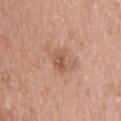biopsy status = total-body-photography surveillance lesion; no biopsy | imaging modality = 15 mm crop, total-body photography | automated lesion analysis = a nevus-likeness score of about 5/100 and a lesion-detection confidence of about 100/100 | lighting = white-light illumination | patient = male, in their 40s | anatomic site = the back | size = ~3 mm (longest diameter).